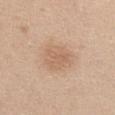No biopsy was performed on this lesion — it was imaged during a full skin examination and was not determined to be concerning.
An algorithmic analysis of the crop reported an area of roughly 10 mm², an outline eccentricity of about 0.65 (0 = round, 1 = elongated), and a shape-asymmetry score of about 0.25 (0 = symmetric). The analysis additionally found a border-irregularity rating of about 2.5/10 and a peripheral color-asymmetry measure near 1.
From the upper back.
Longest diameter approximately 4 mm.
A lesion tile, about 15 mm wide, cut from a 3D total-body photograph.
A female subject, in their 30s.
Captured under white-light illumination.